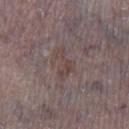The lesion was photographed on a routine skin check and not biopsied; there is no pathology result.
This is a white-light tile.
The lesion-visualizer software estimated a footprint of about 5.5 mm² and a symmetry-axis asymmetry near 0.6. The software also gave an average lesion color of about L≈43 a*≈15 b*≈17 (CIELAB), about 5 CIELAB-L* units darker than the surrounding skin, and a lesion-to-skin contrast of about 5 (normalized; higher = more distinct). And it measured a border-irregularity index near 7/10, internal color variation of about 2 on a 0–10 scale, and peripheral color asymmetry of about 0.5.
The lesion is on the left lower leg.
A male subject, aged 73–77.
A region of skin cropped from a whole-body photographic capture, roughly 15 mm wide.